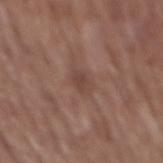Captured during whole-body skin photography for melanoma surveillance; the lesion was not biopsied.
The lesion's longest dimension is about 2.5 mm.
Cropped from a total-body skin-imaging series; the visible field is about 15 mm.
An algorithmic analysis of the crop reported roughly 7 lightness units darker than nearby skin. It also reported a classifier nevus-likeness of about 0/100.
This is a white-light tile.
Located on the mid back.
A male subject in their mid- to late 70s.